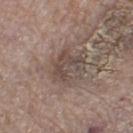Part of a total-body skin-imaging series; this lesion was reviewed on a skin check and was not flagged for biopsy. Automated image analysis of the tile measured an average lesion color of about L≈46 a*≈14 b*≈20 (CIELAB), about 8 CIELAB-L* units darker than the surrounding skin, and a normalized border contrast of about 6.5. A male patient aged 73 to 77. The lesion is on the left thigh. Captured under white-light illumination. A close-up tile cropped from a whole-body skin photograph, about 15 mm across. The lesion's longest dimension is about 4 mm.